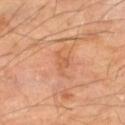Captured during whole-body skin photography for melanoma surveillance; the lesion was not biopsied.
A roughly 15 mm field-of-view crop from a total-body skin photograph.
Located on the left thigh.
A male patient roughly 70 years of age.
Measured at roughly 3 mm in maximum diameter.
Imaged with cross-polarized lighting.